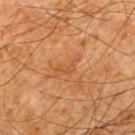Impression:
Imaged during a routine full-body skin examination; the lesion was not biopsied and no histopathology is available.
Context:
Automated tile analysis of the lesion measured a lesion–skin lightness drop of about 5. This image is a 15 mm lesion crop taken from a total-body photograph. The patient is a male aged around 65. Longest diameter approximately 3.5 mm. The lesion is on the upper back. The tile uses cross-polarized illumination.